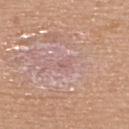Q: Is there a histopathology result?
A: no biopsy performed (imaged during a skin exam)
Q: What is the anatomic site?
A: the upper back
Q: What are the patient's age and sex?
A: male, aged around 40
Q: What kind of image is this?
A: ~15 mm tile from a whole-body skin photo